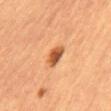Q: Is there a histopathology result?
A: imaged on a skin check; not biopsied
Q: What is the imaging modality?
A: ~15 mm crop, total-body skin-cancer survey
Q: What lighting was used for the tile?
A: cross-polarized illumination
Q: Who is the patient?
A: male, aged approximately 60
Q: What is the anatomic site?
A: the abdomen
Q: What did automated image analysis measure?
A: an area of roughly 4.5 mm², a shape eccentricity near 0.8, and a symmetry-axis asymmetry near 0.15; a border-irregularity index near 1.5/10, a color-variation rating of about 3.5/10, and a peripheral color-asymmetry measure near 1; an automated nevus-likeness rating near 100 out of 100 and lesion-presence confidence of about 100/100
Q: How large is the lesion?
A: ~3 mm (longest diameter)